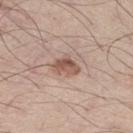subject = male, about 60 years old | image-analysis metrics = a footprint of about 5.5 mm², a shape eccentricity near 0.8, and a symmetry-axis asymmetry near 0.45; a border-irregularity index near 4/10, a within-lesion color-variation index near 4.5/10, and radial color variation of about 1.5 | image source = total-body-photography crop, ~15 mm field of view | diameter = ≈3.5 mm | site = the right thigh | tile lighting = white-light.